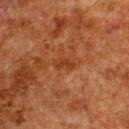biopsy status=catalogued during a skin exam; not biopsied | body site=the upper back | patient=male, approximately 60 years of age | image=~15 mm crop, total-body skin-cancer survey | illumination=cross-polarized illumination | lesion diameter=≈3 mm.